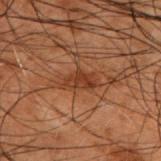Recorded during total-body skin imaging; not selected for excision or biopsy.
This is a cross-polarized tile.
From the upper back.
Automated image analysis of the tile measured a lesion area of about 5 mm² and an outline eccentricity of about 0.85 (0 = round, 1 = elongated). And it measured an automated nevus-likeness rating near 0 out of 100 and a detector confidence of about 95 out of 100 that the crop contains a lesion.
A male subject in their 50s.
A lesion tile, about 15 mm wide, cut from a 3D total-body photograph.